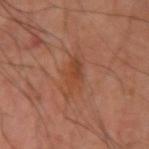Q: Is there a histopathology result?
A: no biopsy performed (imaged during a skin exam)
Q: What kind of image is this?
A: total-body-photography crop, ~15 mm field of view
Q: How was the tile lit?
A: cross-polarized illumination
Q: What is the lesion's diameter?
A: ~4 mm (longest diameter)
Q: What is the anatomic site?
A: the left upper arm
Q: Who is the patient?
A: male, roughly 40 years of age